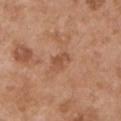The lesion is on the left upper arm.
The patient is a male aged 53–57.
A 15 mm close-up tile from a total-body photography series done for melanoma screening.
An algorithmic analysis of the crop reported a lesion area of about 3.5 mm², a shape eccentricity near 0.8, and a symmetry-axis asymmetry near 0.35. It also reported a border-irregularity index near 3.5/10 and radial color variation of about 0.5. It also reported an automated nevus-likeness rating near 0 out of 100.
The tile uses white-light illumination.